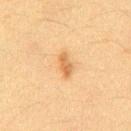<lesion>
  <site>abdomen</site>
  <patient>
    <sex>male</sex>
    <age_approx>35</age_approx>
  </patient>
  <image>
    <source>total-body photography crop</source>
    <field_of_view_mm>15</field_of_view_mm>
  </image>
</lesion>